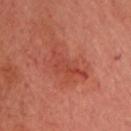Impression: Imaged during a routine full-body skin examination; the lesion was not biopsied and no histopathology is available. Clinical summary: Approximately 5.5 mm at its widest. Located on the head or neck. An algorithmic analysis of the crop reported an area of roughly 8.5 mm², an outline eccentricity of about 0.9 (0 = round, 1 = elongated), and a symmetry-axis asymmetry near 0.5. The analysis additionally found an average lesion color of about L≈45 a*≈32 b*≈31 (CIELAB), roughly 7 lightness units darker than nearby skin, and a normalized border contrast of about 5.5. The software also gave a border-irregularity rating of about 7/10 and a color-variation rating of about 2.5/10. A male subject aged 68 to 72. Captured under cross-polarized illumination. Cropped from a total-body skin-imaging series; the visible field is about 15 mm.